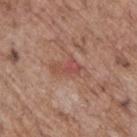Notes:
* notes · catalogued during a skin exam; not biopsied
* location · the upper back
* image · total-body-photography crop, ~15 mm field of view
* tile lighting · white-light
* subject · male, aged approximately 70